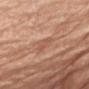Q: Was this lesion biopsied?
A: catalogued during a skin exam; not biopsied
Q: How was this image acquired?
A: total-body-photography crop, ~15 mm field of view
Q: Who is the patient?
A: female, aged around 70
Q: How was the tile lit?
A: white-light illumination
Q: Automated lesion metrics?
A: an eccentricity of roughly 0.7 and two-axis asymmetry of about 0.3; a lesion color around L≈56 a*≈22 b*≈30 in CIELAB and roughly 7 lightness units darker than nearby skin; a classifier nevus-likeness of about 0/100 and lesion-presence confidence of about 95/100
Q: Where on the body is the lesion?
A: the right upper arm